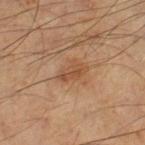This lesion was catalogued during total-body skin photography and was not selected for biopsy.
The lesion is on the leg.
Captured under cross-polarized illumination.
A male patient roughly 60 years of age.
A lesion tile, about 15 mm wide, cut from a 3D total-body photograph.
About 3.5 mm across.